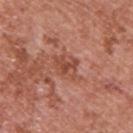Assessment:
Captured during whole-body skin photography for melanoma surveillance; the lesion was not biopsied.
Background:
Cropped from a whole-body photographic skin survey; the tile spans about 15 mm. The lesion's longest dimension is about 4 mm. From the back. An algorithmic analysis of the crop reported an outline eccentricity of about 0.75 (0 = round, 1 = elongated) and two-axis asymmetry of about 0.3. This is a white-light tile. A male subject aged around 70.